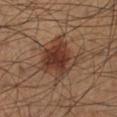Clinical impression:
Recorded during total-body skin imaging; not selected for excision or biopsy.
Clinical summary:
Captured under cross-polarized illumination. A lesion tile, about 15 mm wide, cut from a 3D total-body photograph. Approximately 4.5 mm at its widest. The lesion is located on the left lower leg. A male subject aged approximately 50.